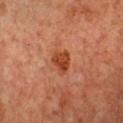notes = no biopsy performed (imaged during a skin exam)
patient = male, aged approximately 60
imaging modality = 15 mm crop, total-body photography
diameter = ~3 mm (longest diameter)
location = the chest
tile lighting = cross-polarized illumination